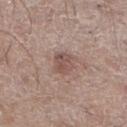workup: no biopsy performed (imaged during a skin exam) | body site: the right lower leg | illumination: white-light illumination | patient: male, about 60 years old | acquisition: ~15 mm tile from a whole-body skin photo | lesion size: about 2.5 mm | image-analysis metrics: a lesion area of about 5 mm², an eccentricity of roughly 0.6, and two-axis asymmetry of about 0.2; a border-irregularity index near 2/10, internal color variation of about 2.5 on a 0–10 scale, and a peripheral color-asymmetry measure near 1; a detector confidence of about 100 out of 100 that the crop contains a lesion.